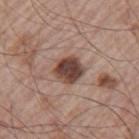Assessment:
Recorded during total-body skin imaging; not selected for excision or biopsy.
Context:
The recorded lesion diameter is about 3.5 mm. A male patient, aged around 65. Automated tile analysis of the lesion measured internal color variation of about 5 on a 0–10 scale and a peripheral color-asymmetry measure near 1.5. Located on the left upper arm. A region of skin cropped from a whole-body photographic capture, roughly 15 mm wide.